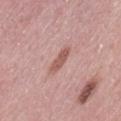Imaged during a routine full-body skin examination; the lesion was not biopsied and no histopathology is available.